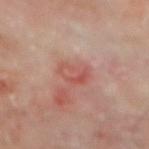Case summary:
- site — the mid back
- subject — male, aged approximately 65
- image source — ~15 mm crop, total-body skin-cancer survey